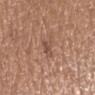location: the arm | subject: female, aged around 55 | image: ~15 mm tile from a whole-body skin photo | lighting: white-light illumination | automated lesion analysis: an outline eccentricity of about 0.8 (0 = round, 1 = elongated) and two-axis asymmetry of about 0.35; lesion-presence confidence of about 100/100 | lesion diameter: ~2.5 mm (longest diameter).